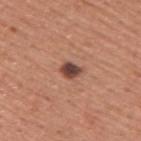notes=total-body-photography surveillance lesion; no biopsy | imaging modality=~15 mm tile from a whole-body skin photo | subject=male, aged 43 to 47 | location=the upper back | lighting=white-light.